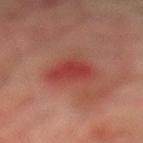Findings:
- anatomic site · the left lower leg
- patient · male, aged around 75
- image · ~15 mm crop, total-body skin-cancer survey